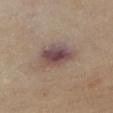Clinical impression: Recorded during total-body skin imaging; not selected for excision or biopsy.